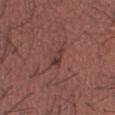– follow-up · no biopsy performed (imaged during a skin exam)
– tile lighting · white-light
– automated lesion analysis · a lesion area of about 2.5 mm², an eccentricity of roughly 0.9, and a shape-asymmetry score of about 0.35 (0 = symmetric); about 8 CIELAB-L* units darker than the surrounding skin and a normalized border contrast of about 7; border irregularity of about 3.5 on a 0–10 scale, internal color variation of about 0 on a 0–10 scale, and peripheral color asymmetry of about 0; a detector confidence of about 75 out of 100 that the crop contains a lesion
– body site · the right lower leg
– subject · male, aged around 45
– image source · ~15 mm crop, total-body skin-cancer survey